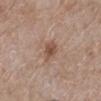Impression: The lesion was photographed on a routine skin check and not biopsied; there is no pathology result. Background: The total-body-photography lesion software estimated an area of roughly 4.5 mm², an outline eccentricity of about 0.6 (0 = round, 1 = elongated), and a shape-asymmetry score of about 0.25 (0 = symmetric). The lesion is on the left lower leg. The subject is a female aged 53–57. Measured at roughly 2.5 mm in maximum diameter. The tile uses white-light illumination. Cropped from a whole-body photographic skin survey; the tile spans about 15 mm.